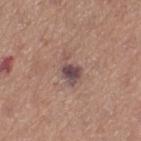The lesion was photographed on a routine skin check and not biopsied; there is no pathology result. The subject is a female aged approximately 55. Cropped from a whole-body photographic skin survey; the tile spans about 15 mm. About 3.5 mm across. The lesion is on the left thigh. Imaged with white-light lighting.